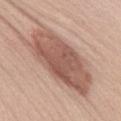* biopsy status — no biopsy performed (imaged during a skin exam)
* imaging modality — ~15 mm crop, total-body skin-cancer survey
* automated lesion analysis — a lesion area of about 37 mm², an eccentricity of roughly 0.9, and a shape-asymmetry score of about 0.2 (0 = symmetric); a peripheral color-asymmetry measure near 1.5; a nevus-likeness score of about 50/100
* location — the mid back
* subject — female, aged 48–52
* illumination — white-light illumination